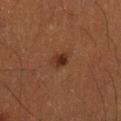Imaged during a routine full-body skin examination; the lesion was not biopsied and no histopathology is available.
About 2.5 mm across.
From the right lower leg.
Captured under cross-polarized illumination.
A 15 mm crop from a total-body photograph taken for skin-cancer surveillance.
A male subject approximately 40 years of age.